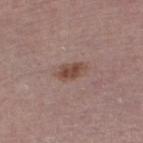{"biopsy_status": "not biopsied; imaged during a skin examination", "image": {"source": "total-body photography crop", "field_of_view_mm": 15}, "patient": {"sex": "female", "age_approx": 70}, "site": "left lower leg"}